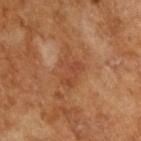Clinical impression: Part of a total-body skin-imaging series; this lesion was reviewed on a skin check and was not flagged for biopsy. Background: A close-up tile cropped from a whole-body skin photograph, about 15 mm across. The patient is a male aged 63 to 67. Longest diameter approximately 5 mm. The tile uses cross-polarized illumination. The lesion-visualizer software estimated a footprint of about 8 mm², an eccentricity of roughly 0.85, and a shape-asymmetry score of about 0.45 (0 = symmetric). The software also gave a lesion color around L≈45 a*≈25 b*≈34 in CIELAB, a lesion–skin lightness drop of about 7, and a lesion-to-skin contrast of about 5.5 (normalized; higher = more distinct). It also reported border irregularity of about 6.5 on a 0–10 scale and a within-lesion color-variation index near 2.5/10. And it measured a nevus-likeness score of about 0/100 and a detector confidence of about 100 out of 100 that the crop contains a lesion.